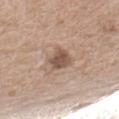Impression:
Imaged during a routine full-body skin examination; the lesion was not biopsied and no histopathology is available.
Background:
Imaged with white-light lighting. A 15 mm close-up tile from a total-body photography series done for melanoma screening. The lesion-visualizer software estimated a mean CIELAB color near L≈53 a*≈17 b*≈26, a lesion–skin lightness drop of about 12, and a normalized border contrast of about 8.5. The software also gave a border-irregularity index near 2.5/10, internal color variation of about 3 on a 0–10 scale, and radial color variation of about 1. And it measured a nevus-likeness score of about 30/100 and lesion-presence confidence of about 100/100. A female subject about 75 years old. Approximately 3 mm at its widest. The lesion is on the arm.